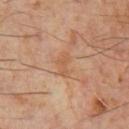Q: Was this lesion biopsied?
A: total-body-photography surveillance lesion; no biopsy
Q: How large is the lesion?
A: about 3 mm
Q: Where on the body is the lesion?
A: the chest
Q: Automated lesion metrics?
A: a border-irregularity rating of about 3.5/10, a within-lesion color-variation index near 1/10, and radial color variation of about 0.5; a classifier nevus-likeness of about 0/100
Q: Who is the patient?
A: male, approximately 60 years of age
Q: Illumination type?
A: cross-polarized
Q: How was this image acquired?
A: ~15 mm tile from a whole-body skin photo